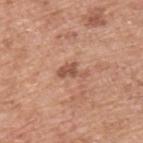Notes:
– notes · total-body-photography surveillance lesion; no biopsy
– image · total-body-photography crop, ~15 mm field of view
– body site · the upper back
– lesion diameter · ~3.5 mm (longest diameter)
– image-analysis metrics · border irregularity of about 5.5 on a 0–10 scale, internal color variation of about 0.5 on a 0–10 scale, and peripheral color asymmetry of about 0; an automated nevus-likeness rating near 0 out of 100
– subject · male, aged 53–57
– lighting · white-light